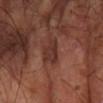biopsy_status: not biopsied; imaged during a skin examination
automated_metrics:
  area_mm2_approx: 7.0
  eccentricity: 0.85
  shape_asymmetry: 0.35
  cielab_L: 32
  cielab_a: 22
  cielab_b: 23
  vs_skin_darker_L: 7.0
  vs_skin_contrast_norm: 7.0
  border_irregularity_0_10: 4.0
  color_variation_0_10: 1.5
  nevus_likeness_0_100: 0
  lesion_detection_confidence_0_100: 70
patient:
  sex: male
  age_approx: 70
image:
  source: total-body photography crop
  field_of_view_mm: 15
lesion_size:
  long_diameter_mm_approx: 4.5
site: right forearm